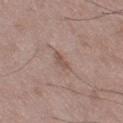| field | value |
|---|---|
| notes | total-body-photography surveillance lesion; no biopsy |
| image source | ~15 mm crop, total-body skin-cancer survey |
| patient | male, aged 48–52 |
| illumination | white-light illumination |
| size | about 2.5 mm |
| anatomic site | the leg |
| automated metrics | a lesion color around L≈52 a*≈17 b*≈24 in CIELAB and about 8 CIELAB-L* units darker than the surrounding skin |